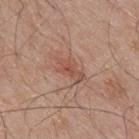Impression: Captured during whole-body skin photography for melanoma surveillance; the lesion was not biopsied. Acquisition and patient details: A male subject aged 68–72. Imaged with white-light lighting. A 15 mm close-up extracted from a 3D total-body photography capture. Approximately 4 mm at its widest. On the upper back.